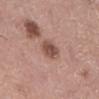follow-up — imaged on a skin check; not biopsied | subject — male, about 50 years old | image source — ~15 mm tile from a whole-body skin photo | illumination — white-light illumination | site — the left lower leg.